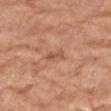Clinical summary:
A lesion tile, about 15 mm wide, cut from a 3D total-body photograph. About 2.5 mm across. The patient is a female about 75 years old. On the right forearm.